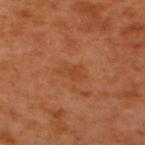Notes:
* acquisition: ~15 mm crop, total-body skin-cancer survey
* anatomic site: the upper back
* lesion diameter: about 3 mm
* subject: male, aged 48–52
* automated metrics: a footprint of about 4.5 mm²; a border-irregularity rating of about 3/10 and a peripheral color-asymmetry measure near 0.5; a nevus-likeness score of about 0/100 and lesion-presence confidence of about 100/100
* lighting: cross-polarized